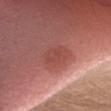notes: total-body-photography surveillance lesion; no biopsy
subject: female, in their 40s
body site: the head or neck
lesion diameter: about 3.5 mm
image: 15 mm crop, total-body photography